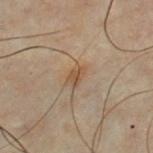Impression: Captured during whole-body skin photography for melanoma surveillance; the lesion was not biopsied. Context: A roughly 15 mm field-of-view crop from a total-body skin photograph. Captured under cross-polarized illumination. About 2.5 mm across. The lesion is located on the chest. The subject is a male in their 50s.